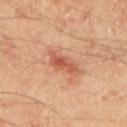Notes:
* notes: imaged on a skin check; not biopsied
* acquisition: 15 mm crop, total-body photography
* lighting: cross-polarized illumination
* subject: male, aged around 65
* automated lesion analysis: a mean CIELAB color near L≈55 a*≈27 b*≈34, about 11 CIELAB-L* units darker than the surrounding skin, and a lesion-to-skin contrast of about 7.5 (normalized; higher = more distinct); an automated nevus-likeness rating near 45 out of 100 and lesion-presence confidence of about 100/100
* lesion size: about 4.5 mm
* body site: the upper back